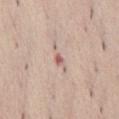Case summary:
* follow-up: total-body-photography surveillance lesion; no biopsy
* lesion diameter: ≈3.5 mm
* location: the abdomen
* subject: female, about 40 years old
* illumination: white-light illumination
* imaging modality: total-body-photography crop, ~15 mm field of view